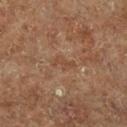The lesion was tiled from a total-body skin photograph and was not biopsied. This is a cross-polarized tile. The lesion-visualizer software estimated a classifier nevus-likeness of about 0/100 and a detector confidence of about 95 out of 100 that the crop contains a lesion. A roughly 15 mm field-of-view crop from a total-body skin photograph. A male subject about 75 years old. Measured at roughly 2.5 mm in maximum diameter. On the left lower leg.